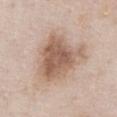The lesion was tiled from a total-body skin photograph and was not biopsied.
A region of skin cropped from a whole-body photographic capture, roughly 15 mm wide.
Automated tile analysis of the lesion measured a lesion–skin lightness drop of about 12 and a normalized lesion–skin contrast near 8. The software also gave a border-irregularity rating of about 4/10, internal color variation of about 5.5 on a 0–10 scale, and peripheral color asymmetry of about 2.
Imaged with white-light lighting.
About 7 mm across.
The subject is a male approximately 55 years of age.
The lesion is located on the chest.